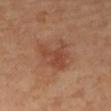Impression: The lesion was tiled from a total-body skin photograph and was not biopsied. Acquisition and patient details: The patient is a female roughly 65 years of age. Approximately 5 mm at its widest. Captured under cross-polarized illumination. The lesion-visualizer software estimated a lesion area of about 9 mm² and two-axis asymmetry of about 0.6. It also reported a classifier nevus-likeness of about 0/100 and a lesion-detection confidence of about 100/100. The lesion is located on the right forearm. A 15 mm close-up extracted from a 3D total-body photography capture.